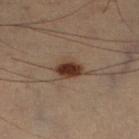Case summary:
* follow-up: total-body-photography surveillance lesion; no biopsy
* image: ~15 mm tile from a whole-body skin photo
* subject: male, aged 53 to 57
* site: the right lower leg